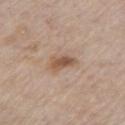Assessment:
Captured during whole-body skin photography for melanoma surveillance; the lesion was not biopsied.
Context:
A region of skin cropped from a whole-body photographic capture, roughly 15 mm wide. A male patient, aged approximately 80. Automated tile analysis of the lesion measured a lesion color around L≈54 a*≈18 b*≈29 in CIELAB, about 11 CIELAB-L* units darker than the surrounding skin, and a lesion-to-skin contrast of about 8 (normalized; higher = more distinct). And it measured a classifier nevus-likeness of about 70/100 and a detector confidence of about 100 out of 100 that the crop contains a lesion. The tile uses white-light illumination. The lesion is located on the chest.